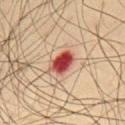{"biopsy_status": "not biopsied; imaged during a skin examination", "patient": {"sex": "male", "age_approx": 65}, "site": "chest", "lesion_size": {"long_diameter_mm_approx": 3.5}, "lighting": "cross-polarized", "image": {"source": "total-body photography crop", "field_of_view_mm": 15}}